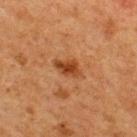{"biopsy_status": "not biopsied; imaged during a skin examination", "patient": {"sex": "male", "age_approx": 65}, "lighting": "cross-polarized", "image": {"source": "total-body photography crop", "field_of_view_mm": 15}, "automated_metrics": {"nevus_likeness_0_100": 80, "lesion_detection_confidence_0_100": 100}, "site": "upper back"}